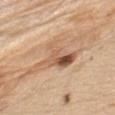<lesion>
  <patient>
    <sex>male</sex>
    <age_approx>70</age_approx>
  </patient>
  <image>
    <source>total-body photography crop</source>
    <field_of_view_mm>15</field_of_view_mm>
  </image>
  <lesion_size>
    <long_diameter_mm_approx>6.0</long_diameter_mm_approx>
  </lesion_size>
  <lighting>white-light</lighting>
  <site>left upper arm</site>
</lesion>